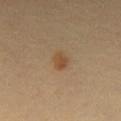biopsy status = imaged on a skin check; not biopsied
image source = 15 mm crop, total-body photography
subject = male, approximately 40 years of age
site = the chest
lesion diameter = about 3 mm
lighting = cross-polarized illumination